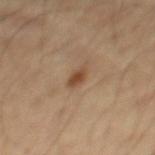The lesion was tiled from a total-body skin photograph and was not biopsied.
Captured under cross-polarized illumination.
A male subject, aged around 55.
This image is a 15 mm lesion crop taken from a total-body photograph.
Longest diameter approximately 2.5 mm.
Located on the back.